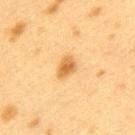<record>
  <biopsy_status>not biopsied; imaged during a skin examination</biopsy_status>
  <patient>
    <sex>female</sex>
    <age_approx>40</age_approx>
  </patient>
  <image>
    <source>total-body photography crop</source>
    <field_of_view_mm>15</field_of_view_mm>
  </image>
  <lesion_size>
    <long_diameter_mm_approx>3.0</long_diameter_mm_approx>
  </lesion_size>
  <lighting>cross-polarized</lighting>
  <site>upper back</site>
</record>